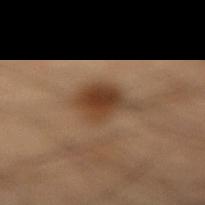A roughly 15 mm field-of-view crop from a total-body skin photograph. This is a cross-polarized tile. Longest diameter approximately 4.5 mm. A male subject, aged 38–42. From the right lower leg.